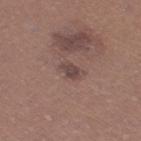{"biopsy_status": "not biopsied; imaged during a skin examination", "image": {"source": "total-body photography crop", "field_of_view_mm": 15}, "site": "right thigh", "patient": {"sex": "female", "age_approx": 40}, "automated_metrics": {"color_variation_0_10": 1.5}, "lighting": "white-light", "lesion_size": {"long_diameter_mm_approx": 2.5}}